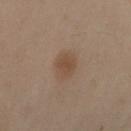| field | value |
|---|---|
| follow-up | catalogued during a skin exam; not biopsied |
| imaging modality | 15 mm crop, total-body photography |
| patient | female, aged approximately 55 |
| body site | the right upper arm |
| size | ≈3 mm |
| lighting | cross-polarized |
| automated lesion analysis | an average lesion color of about L≈48 a*≈16 b*≈28 (CIELAB); a border-irregularity index near 2/10, internal color variation of about 2 on a 0–10 scale, and peripheral color asymmetry of about 0.5 |